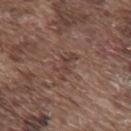Q: Was this lesion biopsied?
A: total-body-photography surveillance lesion; no biopsy
Q: What lighting was used for the tile?
A: white-light
Q: What did automated image analysis measure?
A: an eccentricity of roughly 0.9 and two-axis asymmetry of about 0.55; about 6 CIELAB-L* units darker than the surrounding skin and a lesion-to-skin contrast of about 5.5 (normalized; higher = more distinct); border irregularity of about 7.5 on a 0–10 scale; a detector confidence of about 95 out of 100 that the crop contains a lesion
Q: Lesion location?
A: the upper back
Q: How was this image acquired?
A: 15 mm crop, total-body photography
Q: What are the patient's age and sex?
A: male, about 75 years old